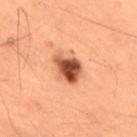Clinical impression: No biopsy was performed on this lesion — it was imaged during a full skin examination and was not determined to be concerning. Image and clinical context: The recorded lesion diameter is about 3.5 mm. A male subject aged approximately 50. Located on the back. A roughly 15 mm field-of-view crop from a total-body skin photograph. Imaged with cross-polarized lighting.